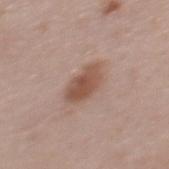biopsy status = catalogued during a skin exam; not biopsied.